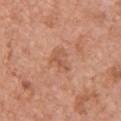Assessment:
Captured during whole-body skin photography for melanoma surveillance; the lesion was not biopsied.
Clinical summary:
The lesion's longest dimension is about 3 mm. From the upper back. The subject is a male approximately 80 years of age. A region of skin cropped from a whole-body photographic capture, roughly 15 mm wide.